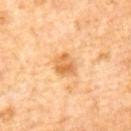notes: imaged on a skin check; not biopsied
TBP lesion metrics: a lesion color around L≈64 a*≈23 b*≈44 in CIELAB and a lesion-to-skin contrast of about 7 (normalized; higher = more distinct)
subject: male, aged 63–67
image: ~15 mm crop, total-body skin-cancer survey
site: the mid back